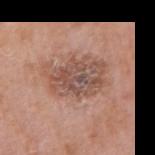Q: Was a biopsy performed?
A: total-body-photography surveillance lesion; no biopsy
Q: What is the lesion's diameter?
A: ~6.5 mm (longest diameter)
Q: Who is the patient?
A: male, about 60 years old
Q: Where on the body is the lesion?
A: the right upper arm
Q: What lighting was used for the tile?
A: white-light illumination
Q: What kind of image is this?
A: total-body-photography crop, ~15 mm field of view